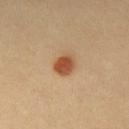Clinical impression: Captured during whole-body skin photography for melanoma surveillance; the lesion was not biopsied.